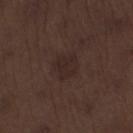{"biopsy_status": "not biopsied; imaged during a skin examination"}